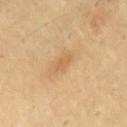On the chest. A 15 mm close-up extracted from a 3D total-body photography capture. A male subject, aged around 55. About 1.5 mm across. Captured under cross-polarized illumination.